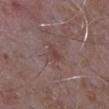Imaged during a routine full-body skin examination; the lesion was not biopsied and no histopathology is available.
The lesion is on the left upper arm.
About 2.5 mm across.
A region of skin cropped from a whole-body photographic capture, roughly 15 mm wide.
This is a white-light tile.
The patient is a male aged 63 to 67.
Automated image analysis of the tile measured a lesion area of about 4.5 mm² and a shape-asymmetry score of about 0.5 (0 = symmetric). And it measured a border-irregularity index near 4.5/10, a color-variation rating of about 2/10, and peripheral color asymmetry of about 0.5. The analysis additionally found a detector confidence of about 100 out of 100 that the crop contains a lesion.